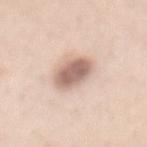Impression:
The lesion was photographed on a routine skin check and not biopsied; there is no pathology result.
Image and clinical context:
The tile uses white-light illumination. Cropped from a total-body skin-imaging series; the visible field is about 15 mm. A female subject in their 40s. On the mid back.